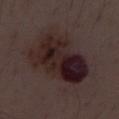<lesion>
<lesion_size>
  <long_diameter_mm_approx>8.0</long_diameter_mm_approx>
</lesion_size>
<image>
  <source>total-body photography crop</source>
  <field_of_view_mm>15</field_of_view_mm>
</image>
<site>front of the torso</site>
<lighting>white-light</lighting>
<patient>
  <sex>male</sex>
  <age_approx>55</age_approx>
</patient>
</lesion>